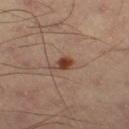The lesion was photographed on a routine skin check and not biopsied; there is no pathology result. A male patient aged around 55. Captured under cross-polarized illumination. Measured at roughly 2.5 mm in maximum diameter. Automated tile analysis of the lesion measured a footprint of about 3.5 mm², an eccentricity of roughly 0.75, and two-axis asymmetry of about 0.3. It also reported a mean CIELAB color near L≈35 a*≈18 b*≈25, roughly 10 lightness units darker than nearby skin, and a normalized border contrast of about 9.5. The analysis additionally found a border-irregularity rating of about 2.5/10, internal color variation of about 2.5 on a 0–10 scale, and radial color variation of about 0.5. The analysis additionally found a nevus-likeness score of about 100/100 and a detector confidence of about 100 out of 100 that the crop contains a lesion. The lesion is located on the left thigh. A lesion tile, about 15 mm wide, cut from a 3D total-body photograph.